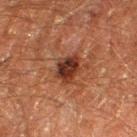Findings:
• biopsy status — total-body-photography surveillance lesion; no biopsy
• automated metrics — a footprint of about 7.5 mm², a shape eccentricity near 0.6, and two-axis asymmetry of about 0.25; about 11 CIELAB-L* units darker than the surrounding skin and a lesion-to-skin contrast of about 11 (normalized; higher = more distinct); a border-irregularity rating of about 2.5/10, internal color variation of about 4 on a 0–10 scale, and radial color variation of about 1; a classifier nevus-likeness of about 75/100 and a lesion-detection confidence of about 100/100
• lighting — cross-polarized
• lesion diameter — ≈3.5 mm
• body site — the right thigh
• patient — male, aged 58–62
• acquisition — 15 mm crop, total-body photography